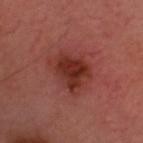biopsy status = total-body-photography surveillance lesion; no biopsy
subject = male, in their 60s
image source = ~15 mm crop, total-body skin-cancer survey
lesion diameter = ~4.5 mm (longest diameter)
lighting = cross-polarized
site = the head or neck